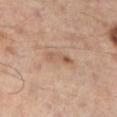Imaged during a routine full-body skin examination; the lesion was not biopsied and no histopathology is available.
The lesion is on the left leg.
The subject is a male about 50 years old.
A 15 mm close-up extracted from a 3D total-body photography capture.